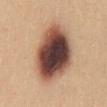A female patient, aged 23–27. A region of skin cropped from a whole-body photographic capture, roughly 15 mm wide. The lesion is located on the mid back. Longest diameter approximately 8.5 mm. Imaged with white-light lighting.